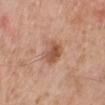Case summary:
- follow-up: imaged on a skin check; not biopsied
- size: ~3 mm (longest diameter)
- TBP lesion metrics: about 11 CIELAB-L* units darker than the surrounding skin and a normalized border contrast of about 7.5
- body site: the left lower leg
- imaging modality: total-body-photography crop, ~15 mm field of view
- lighting: white-light illumination
- subject: male, roughly 80 years of age